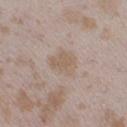Q: Was this lesion biopsied?
A: no biopsy performed (imaged during a skin exam)
Q: What lighting was used for the tile?
A: white-light
Q: What are the patient's age and sex?
A: female, about 25 years old
Q: What is the anatomic site?
A: the leg
Q: What kind of image is this?
A: total-body-photography crop, ~15 mm field of view
Q: Automated lesion metrics?
A: a lesion area of about 7 mm², an outline eccentricity of about 0.6 (0 = round, 1 = elongated), and a shape-asymmetry score of about 0.3 (0 = symmetric); an average lesion color of about L≈59 a*≈13 b*≈26 (CIELAB), a lesion–skin lightness drop of about 6, and a lesion-to-skin contrast of about 5.5 (normalized; higher = more distinct); an automated nevus-likeness rating near 10 out of 100 and a lesion-detection confidence of about 100/100